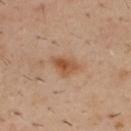Captured during whole-body skin photography for melanoma surveillance; the lesion was not biopsied. The lesion is on the upper back. A male subject in their 40s. A region of skin cropped from a whole-body photographic capture, roughly 15 mm wide. Automated image analysis of the tile measured an outline eccentricity of about 0.75 (0 = round, 1 = elongated) and a symmetry-axis asymmetry near 0.4. The analysis additionally found an average lesion color of about L≈50 a*≈22 b*≈34 (CIELAB), roughly 10 lightness units darker than nearby skin, and a normalized lesion–skin contrast near 8.5. And it measured internal color variation of about 4 on a 0–10 scale and peripheral color asymmetry of about 1.5.